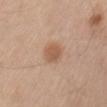size = ~3 mm (longest diameter); image = ~15 mm tile from a whole-body skin photo; subject = male, aged approximately 60; anatomic site = the lower back; tile lighting = white-light illumination.